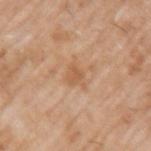Q: Was a biopsy performed?
A: no biopsy performed (imaged during a skin exam)
Q: What did automated image analysis measure?
A: a border-irregularity index near 4/10, a within-lesion color-variation index near 1.5/10, and radial color variation of about 0.5; a detector confidence of about 100 out of 100 that the crop contains a lesion
Q: Patient demographics?
A: male, approximately 60 years of age
Q: Lesion location?
A: the arm
Q: What is the lesion's diameter?
A: ≈2.5 mm
Q: What is the imaging modality?
A: total-body-photography crop, ~15 mm field of view